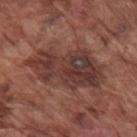| key | value |
|---|---|
| follow-up | total-body-photography surveillance lesion; no biopsy |
| image source | 15 mm crop, total-body photography |
| illumination | white-light illumination |
| subject | male, aged 73 to 77 |
| size | about 9 mm |
| location | the right upper arm |